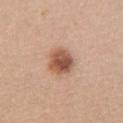Recorded during total-body skin imaging; not selected for excision or biopsy. The lesion is located on the chest. A 15 mm crop from a total-body photograph taken for skin-cancer surveillance. The subject is a female aged approximately 30. About 3.5 mm across.